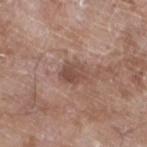| key | value |
|---|---|
| workup | catalogued during a skin exam; not biopsied |
| image source | ~15 mm tile from a whole-body skin photo |
| subject | male, aged 58–62 |
| tile lighting | white-light illumination |
| lesion size | ≈3.5 mm |
| anatomic site | the left lower leg |